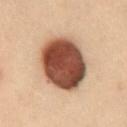Findings:
* patient: female, roughly 30 years of age
* site: the back
* imaging modality: 15 mm crop, total-body photography